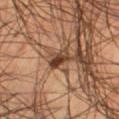Clinical impression:
The lesion was photographed on a routine skin check and not biopsied; there is no pathology result.
Acquisition and patient details:
A 15 mm close-up tile from a total-body photography series done for melanoma screening. A male patient, aged 43–47. The lesion's longest dimension is about 3.5 mm. The tile uses cross-polarized illumination. On the right thigh.